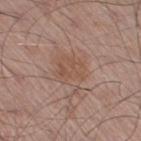Q: Lesion size?
A: ≈5 mm
Q: What did automated image analysis measure?
A: an area of roughly 12 mm², a shape eccentricity near 0.65, and a symmetry-axis asymmetry near 0.3; a nevus-likeness score of about 5/100 and lesion-presence confidence of about 100/100
Q: What is the imaging modality?
A: ~15 mm tile from a whole-body skin photo
Q: Illumination type?
A: white-light illumination
Q: What are the patient's age and sex?
A: male, aged approximately 55
Q: Lesion location?
A: the leg